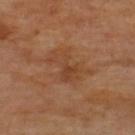Imaged during a routine full-body skin examination; the lesion was not biopsied and no histopathology is available.
A 15 mm crop from a total-body photograph taken for skin-cancer surveillance.
A subject in their mid-60s.
Measured at roughly 5 mm in maximum diameter.
An algorithmic analysis of the crop reported a normalized lesion–skin contrast near 5.5. And it measured internal color variation of about 3.5 on a 0–10 scale and radial color variation of about 1. And it measured a classifier nevus-likeness of about 0/100.
From the upper back.